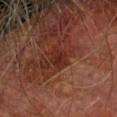Q: Is there a histopathology result?
A: catalogued during a skin exam; not biopsied
Q: What did automated image analysis measure?
A: a footprint of about 4.5 mm², an outline eccentricity of about 0.75 (0 = round, 1 = elongated), and a symmetry-axis asymmetry near 0.3; a lesion color around L≈22 a*≈21 b*≈22 in CIELAB; an automated nevus-likeness rating near 10 out of 100 and lesion-presence confidence of about 100/100
Q: Patient demographics?
A: male, aged 73 to 77
Q: How was the tile lit?
A: cross-polarized
Q: What is the imaging modality?
A: ~15 mm crop, total-body skin-cancer survey
Q: How large is the lesion?
A: ~3 mm (longest diameter)
Q: What is the anatomic site?
A: the left forearm